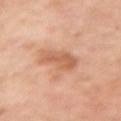Notes:
* follow-up — catalogued during a skin exam; not biopsied